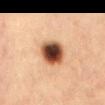Impression: No biopsy was performed on this lesion — it was imaged during a full skin examination and was not determined to be concerning. Context: Measured at roughly 4 mm in maximum diameter. Automated image analysis of the tile measured a mean CIELAB color near L≈40 a*≈20 b*≈28 and a lesion–skin lightness drop of about 21. The analysis additionally found border irregularity of about 1.5 on a 0–10 scale, a color-variation rating of about 9/10, and a peripheral color-asymmetry measure near 2.5. The software also gave an automated nevus-likeness rating near 100 out of 100 and a lesion-detection confidence of about 100/100. A roughly 15 mm field-of-view crop from a total-body skin photograph. On the abdomen. This is a cross-polarized tile. The subject is a female aged approximately 40.